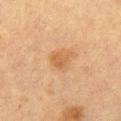biopsy status: total-body-photography surveillance lesion; no biopsy
anatomic site: the left thigh
lesion size: ≈2.5 mm
patient: female, approximately 55 years of age
image source: 15 mm crop, total-body photography